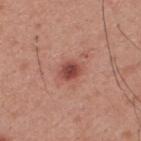A 15 mm close-up extracted from a 3D total-body photography capture.
A male patient, aged 28 to 32.
The lesion is located on the upper back.